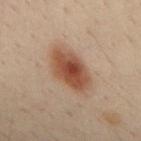Clinical impression: Imaged during a routine full-body skin examination; the lesion was not biopsied and no histopathology is available. Acquisition and patient details: A male patient, approximately 30 years of age. A 15 mm close-up extracted from a 3D total-body photography capture. On the mid back.